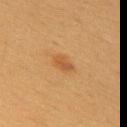biopsy status: total-body-photography surveillance lesion; no biopsy
subject: female, aged 18–22
anatomic site: the upper back
tile lighting: cross-polarized illumination
size: ≈2.5 mm
image: ~15 mm tile from a whole-body skin photo